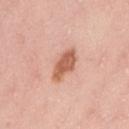Clinical impression:
Part of a total-body skin-imaging series; this lesion was reviewed on a skin check and was not flagged for biopsy.
Context:
An algorithmic analysis of the crop reported an area of roughly 8 mm² and two-axis asymmetry of about 0.2. The software also gave a border-irregularity index near 2/10, a color-variation rating of about 3/10, and a peripheral color-asymmetry measure near 1. From the right upper arm. Cropped from a total-body skin-imaging series; the visible field is about 15 mm. Imaged with white-light lighting. A female subject, approximately 25 years of age. The recorded lesion diameter is about 4 mm.